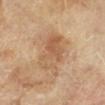Q: Was this lesion biopsied?
A: total-body-photography surveillance lesion; no biopsy
Q: How large is the lesion?
A: about 6.5 mm
Q: Illumination type?
A: cross-polarized
Q: Patient demographics?
A: male, in their mid-60s
Q: What is the anatomic site?
A: the right lower leg
Q: What is the imaging modality?
A: 15 mm crop, total-body photography